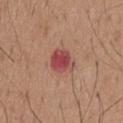Case summary:
– workup: no biopsy performed (imaged during a skin exam)